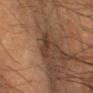{"biopsy_status": "not biopsied; imaged during a skin examination", "automated_metrics": {"cielab_L": 30, "cielab_a": 14, "cielab_b": 23, "vs_skin_contrast_norm": 7.0, "border_irregularity_0_10": 3.0, "color_variation_0_10": 2.0, "peripheral_color_asymmetry": 1.0, "nevus_likeness_0_100": 20, "lesion_detection_confidence_0_100": 80}, "image": {"source": "total-body photography crop", "field_of_view_mm": 15}, "site": "left lower leg", "patient": {"sex": "male", "age_approx": 60}}